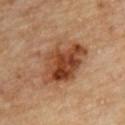follow-up: no biopsy performed (imaged during a skin exam)
patient: male, aged 83 or older
anatomic site: the upper back
image source: 15 mm crop, total-body photography
illumination: cross-polarized